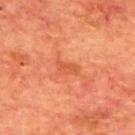Part of a total-body skin-imaging series; this lesion was reviewed on a skin check and was not flagged for biopsy.
Located on the upper back.
A roughly 15 mm field-of-view crop from a total-body skin photograph.
Imaged with cross-polarized lighting.
A male subject in their mid- to late 60s.
An algorithmic analysis of the crop reported a footprint of about 3 mm², an eccentricity of roughly 0.85, and two-axis asymmetry of about 0.4. And it measured border irregularity of about 3.5 on a 0–10 scale, internal color variation of about 1 on a 0–10 scale, and radial color variation of about 0.5. The analysis additionally found a nevus-likeness score of about 0/100 and a lesion-detection confidence of about 100/100.